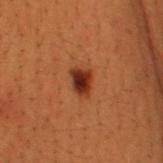Assessment: This lesion was catalogued during total-body skin photography and was not selected for biopsy. Context: Measured at roughly 2.5 mm in maximum diameter. Automated tile analysis of the lesion measured an average lesion color of about L≈26 a*≈24 b*≈28 (CIELAB) and roughly 13 lightness units darker than nearby skin. And it measured a border-irregularity rating of about 2.5/10, a within-lesion color-variation index near 5/10, and radial color variation of about 1.5. It also reported a nevus-likeness score of about 100/100. A male subject aged 48–52. A lesion tile, about 15 mm wide, cut from a 3D total-body photograph. From the head or neck.